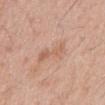The lesion was photographed on a routine skin check and not biopsied; there is no pathology result.
On the abdomen.
Imaged with white-light lighting.
A lesion tile, about 15 mm wide, cut from a 3D total-body photograph.
The total-body-photography lesion software estimated an eccentricity of roughly 0.9 and a symmetry-axis asymmetry near 0.3. The analysis additionally found an average lesion color of about L≈61 a*≈21 b*≈31 (CIELAB), a lesion–skin lightness drop of about 7, and a normalized border contrast of about 5. And it measured a color-variation rating of about 2.5/10 and a peripheral color-asymmetry measure near 1.
A male patient, in their mid-70s.